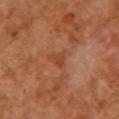{
  "biopsy_status": "not biopsied; imaged during a skin examination",
  "image": {
    "source": "total-body photography crop",
    "field_of_view_mm": 15
  },
  "lighting": "cross-polarized",
  "automated_metrics": {
    "cielab_L": 44,
    "cielab_a": 28,
    "cielab_b": 35,
    "vs_skin_darker_L": 7.0,
    "vs_skin_contrast_norm": 5.5
  },
  "site": "chest",
  "lesion_size": {
    "long_diameter_mm_approx": 1.5
  },
  "patient": {
    "sex": "female",
    "age_approx": 55
  }
}